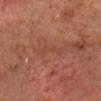Q: Is there a histopathology result?
A: total-body-photography surveillance lesion; no biopsy
Q: How was the tile lit?
A: cross-polarized
Q: Lesion location?
A: the front of the torso
Q: Lesion size?
A: ~3.5 mm (longest diameter)
Q: What are the patient's age and sex?
A: male, aged approximately 65
Q: How was this image acquired?
A: total-body-photography crop, ~15 mm field of view